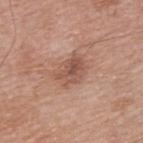follow-up = no biopsy performed (imaged during a skin exam) | patient = male, roughly 40 years of age | acquisition = 15 mm crop, total-body photography | size = about 4 mm | tile lighting = white-light illumination | site = the back.